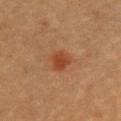Impression: The lesion was photographed on a routine skin check and not biopsied; there is no pathology result. Acquisition and patient details: A roughly 15 mm field-of-view crop from a total-body skin photograph. The total-body-photography lesion software estimated an eccentricity of roughly 0.55 and a symmetry-axis asymmetry near 0.25. And it measured roughly 8 lightness units darker than nearby skin and a lesion-to-skin contrast of about 7.5 (normalized; higher = more distinct). The software also gave a border-irregularity index near 2.5/10, a color-variation rating of about 2.5/10, and a peripheral color-asymmetry measure near 1. The lesion is located on the chest. A female subject, roughly 60 years of age. Imaged with cross-polarized lighting. The recorded lesion diameter is about 2.5 mm.